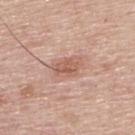The lesion was tiled from a total-body skin photograph and was not biopsied.
Captured under white-light illumination.
Cropped from a total-body skin-imaging series; the visible field is about 15 mm.
The patient is a male in their mid- to late 50s.
On the upper back.
About 3.5 mm across.